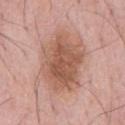Clinical impression:
Part of a total-body skin-imaging series; this lesion was reviewed on a skin check and was not flagged for biopsy.
Context:
A male patient aged around 60. About 8 mm across. From the chest. Automated image analysis of the tile measured an area of roughly 31 mm², an outline eccentricity of about 0.75 (0 = round, 1 = elongated), and a symmetry-axis asymmetry near 0.2. It also reported a border-irregularity rating of about 3/10, a color-variation rating of about 5/10, and radial color variation of about 1.5. It also reported a classifier nevus-likeness of about 50/100. Cropped from a whole-body photographic skin survey; the tile spans about 15 mm.